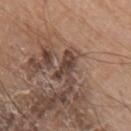- follow-up · total-body-photography surveillance lesion; no biopsy
- location · the arm
- patient · male, about 80 years old
- illumination · white-light illumination
- image · ~15 mm crop, total-body skin-cancer survey